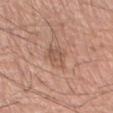Acquisition and patient details: A male subject aged 53–57. Automated tile analysis of the lesion measured a mean CIELAB color near L≈55 a*≈21 b*≈28 and roughly 8 lightness units darker than nearby skin. This image is a 15 mm lesion crop taken from a total-body photograph. The lesion is located on the right forearm.